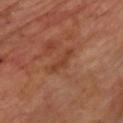{
  "biopsy_status": "not biopsied; imaged during a skin examination",
  "lesion_size": {
    "long_diameter_mm_approx": 3.5
  },
  "automated_metrics": {
    "border_irregularity_0_10": 6.5,
    "color_variation_0_10": 0.0,
    "peripheral_color_asymmetry": 0.0
  },
  "patient": {
    "sex": "male",
    "age_approx": 70
  },
  "site": "chest",
  "image": {
    "source": "total-body photography crop",
    "field_of_view_mm": 15
  }
}